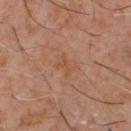Clinical impression: No biopsy was performed on this lesion — it was imaged during a full skin examination and was not determined to be concerning. Image and clinical context: Cropped from a whole-body photographic skin survey; the tile spans about 15 mm. The tile uses cross-polarized illumination. On the chest. Automated image analysis of the tile measured a footprint of about 4.5 mm², an outline eccentricity of about 0.65 (0 = round, 1 = elongated), and a symmetry-axis asymmetry near 0.3. The analysis additionally found an average lesion color of about L≈47 a*≈19 b*≈31 (CIELAB), a lesion–skin lightness drop of about 4, and a normalized border contrast of about 5. The software also gave a border-irregularity rating of about 4/10, a within-lesion color-variation index near 2.5/10, and radial color variation of about 1. And it measured lesion-presence confidence of about 100/100. About 3 mm across. The subject is a male about 60 years old.